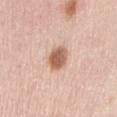Recorded during total-body skin imaging; not selected for excision or biopsy.
The lesion is located on the abdomen.
This is a white-light tile.
A female subject in their mid- to late 60s.
The recorded lesion diameter is about 3 mm.
Cropped from a total-body skin-imaging series; the visible field is about 15 mm.